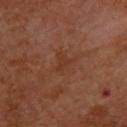Clinical impression:
Recorded during total-body skin imaging; not selected for excision or biopsy.